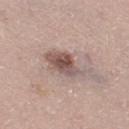The lesion was photographed on a routine skin check and not biopsied; there is no pathology result.
The recorded lesion diameter is about 6 mm.
Imaged with white-light lighting.
A close-up tile cropped from a whole-body skin photograph, about 15 mm across.
A female subject aged around 40.
On the leg.